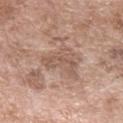Q: Was a biopsy performed?
A: catalogued during a skin exam; not biopsied
Q: Automated lesion metrics?
A: a color-variation rating of about 2/10 and peripheral color asymmetry of about 0.5
Q: Who is the patient?
A: female, approximately 75 years of age
Q: Illumination type?
A: white-light illumination
Q: What is the anatomic site?
A: the right forearm
Q: How large is the lesion?
A: about 4.5 mm
Q: What kind of image is this?
A: 15 mm crop, total-body photography